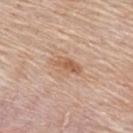Part of a total-body skin-imaging series; this lesion was reviewed on a skin check and was not flagged for biopsy. A close-up tile cropped from a whole-body skin photograph, about 15 mm across. Located on the upper back. Approximately 4 mm at its widest. A female patient aged 63 to 67.